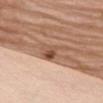biopsy status: total-body-photography surveillance lesion; no biopsy
anatomic site: the chest
imaging modality: total-body-photography crop, ~15 mm field of view
size: ≈5 mm
subject: female, approximately 75 years of age
lighting: white-light